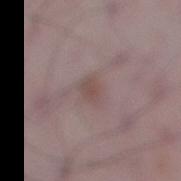Q: Is there a histopathology result?
A: imaged on a skin check; not biopsied
Q: What is the anatomic site?
A: the leg
Q: How was this image acquired?
A: ~15 mm tile from a whole-body skin photo
Q: Illumination type?
A: white-light
Q: What is the lesion's diameter?
A: ≈2.5 mm
Q: Who is the patient?
A: male, aged approximately 50